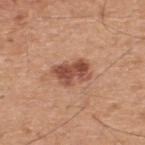No biopsy was performed on this lesion — it was imaged during a full skin examination and was not determined to be concerning. The recorded lesion diameter is about 4 mm. The lesion-visualizer software estimated roughly 13 lightness units darker than nearby skin. The lesion is located on the upper back. A 15 mm close-up extracted from a 3D total-body photography capture. A male patient, approximately 45 years of age.